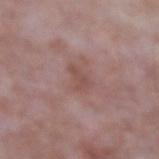Assessment:
The lesion was tiled from a total-body skin photograph and was not biopsied.
Background:
A male patient aged 63–67. A 15 mm close-up extracted from a 3D total-body photography capture. On the left forearm. The tile uses white-light illumination. The lesion-visualizer software estimated an area of roughly 4 mm² and an eccentricity of roughly 0.75. Longest diameter approximately 2.5 mm.